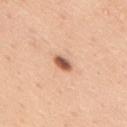* workup — total-body-photography surveillance lesion; no biopsy
* lesion size — ~2.5 mm (longest diameter)
* image — ~15 mm tile from a whole-body skin photo
* site — the back
* patient — male, in their mid- to late 30s
* tile lighting — white-light